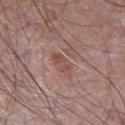Clinical impression: No biopsy was performed on this lesion — it was imaged during a full skin examination and was not determined to be concerning. Context: Captured under white-light illumination. On the chest. A 15 mm close-up extracted from a 3D total-body photography capture. A male patient, aged around 70. About 3 mm across.